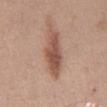notes = total-body-photography surveillance lesion; no biopsy | image = 15 mm crop, total-body photography | site = the chest | subject = female, aged approximately 50 | lighting = white-light | image-analysis metrics = a nevus-likeness score of about 60/100 | size = ~7 mm (longest diameter).